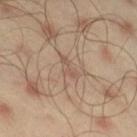{
  "biopsy_status": "not biopsied; imaged during a skin examination",
  "site": "right thigh",
  "automated_metrics": {
    "vs_skin_darker_L": 7.0,
    "border_irregularity_0_10": 5.5,
    "color_variation_0_10": 0.0,
    "nevus_likeness_0_100": 0,
    "lesion_detection_confidence_0_100": 85
  },
  "image": {
    "source": "total-body photography crop",
    "field_of_view_mm": 15
  },
  "patient": {
    "sex": "male",
    "age_approx": 45
  },
  "lesion_size": {
    "long_diameter_mm_approx": 3.0
  }
}